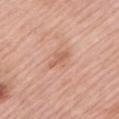No biopsy was performed on this lesion — it was imaged during a full skin examination and was not determined to be concerning.
A male subject, aged approximately 75.
A lesion tile, about 15 mm wide, cut from a 3D total-body photograph.
Located on the left upper arm.